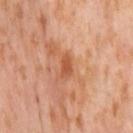Impression:
Captured during whole-body skin photography for melanoma surveillance; the lesion was not biopsied.
Background:
A female patient, aged 53 to 57. This image is a 15 mm lesion crop taken from a total-body photograph. The lesion's longest dimension is about 2.5 mm. Automated image analysis of the tile measured a footprint of about 3 mm², a shape eccentricity near 0.85, and a symmetry-axis asymmetry near 0.25. And it measured a nevus-likeness score of about 0/100 and a lesion-detection confidence of about 100/100. From the right thigh.